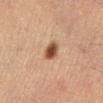workup: total-body-photography surveillance lesion; no biopsy | acquisition: 15 mm crop, total-body photography | patient: female, aged around 55 | anatomic site: the right lower leg | lighting: cross-polarized.